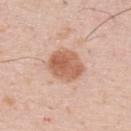Part of a total-body skin-imaging series; this lesion was reviewed on a skin check and was not flagged for biopsy. A male subject, approximately 35 years of age. A roughly 15 mm field-of-view crop from a total-body skin photograph. On the upper back.